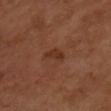Recorded during total-body skin imaging; not selected for excision or biopsy.
A 15 mm crop from a total-body photograph taken for skin-cancer surveillance.
A male patient, aged 63–67.
Imaged with cross-polarized lighting.
An algorithmic analysis of the crop reported an area of roughly 3.5 mm², an eccentricity of roughly 0.9, and two-axis asymmetry of about 0.5. The software also gave border irregularity of about 4.5 on a 0–10 scale and peripheral color asymmetry of about 0.5.